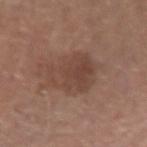Assessment: Imaged during a routine full-body skin examination; the lesion was not biopsied and no histopathology is available. Image and clinical context: The lesion is on the left forearm. Imaged with white-light lighting. Approximately 6 mm at its widest. A female subject, roughly 50 years of age. The total-body-photography lesion software estimated an area of roughly 15 mm², an eccentricity of roughly 0.75, and two-axis asymmetry of about 0.25. It also reported a lesion color around L≈43 a*≈19 b*≈25 in CIELAB, a lesion–skin lightness drop of about 8, and a lesion-to-skin contrast of about 6.5 (normalized; higher = more distinct). It also reported a classifier nevus-likeness of about 45/100. Cropped from a whole-body photographic skin survey; the tile spans about 15 mm.